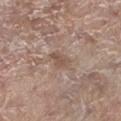notes — imaged on a skin check; not biopsied | acquisition — 15 mm crop, total-body photography | site — the left lower leg | subject — female, roughly 85 years of age or older.